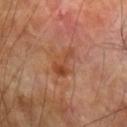  biopsy_status: not biopsied; imaged during a skin examination
  patient:
    sex: male
    age_approx: 70
  site: arm
  lesion_size:
    long_diameter_mm_approx: 4.0
  automated_metrics:
    area_mm2_approx: 7.0
    shape_asymmetry: 0.4
    nevus_likeness_0_100: 0
    lesion_detection_confidence_0_100: 100
  lighting: cross-polarized
  image:
    source: total-body photography crop
    field_of_view_mm: 15